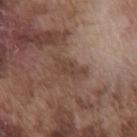Clinical impression:
This lesion was catalogued during total-body skin photography and was not selected for biopsy.
Background:
A male subject roughly 75 years of age. Located on the chest. A close-up tile cropped from a whole-body skin photograph, about 15 mm across. The lesion's longest dimension is about 4 mm. The tile uses white-light illumination.